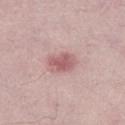No biopsy was performed on this lesion — it was imaged during a full skin examination and was not determined to be concerning.
Automated tile analysis of the lesion measured an area of roughly 7 mm², an outline eccentricity of about 0.75 (0 = round, 1 = elongated), and two-axis asymmetry of about 0.2.
The patient is a male in their 30s.
Captured under white-light illumination.
Cropped from a whole-body photographic skin survey; the tile spans about 15 mm.
Measured at roughly 3.5 mm in maximum diameter.